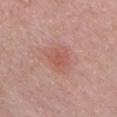  biopsy_status: not biopsied; imaged during a skin examination
  automated_metrics:
    border_irregularity_0_10: 2.0
    color_variation_0_10: 2.5
    peripheral_color_asymmetry: 0.5
    nevus_likeness_0_100: 65
    lesion_detection_confidence_0_100: 100
  image:
    source: total-body photography crop
    field_of_view_mm: 15
  site: chest
  lighting: white-light
  patient:
    sex: male
    age_approx: 50